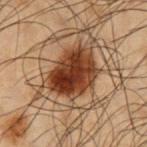Notes:
- follow-up: catalogued during a skin exam; not biopsied
- site: the right upper arm
- image: ~15 mm tile from a whole-body skin photo
- subject: male, about 50 years old